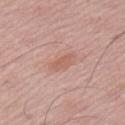The lesion was tiled from a total-body skin photograph and was not biopsied. Captured under white-light illumination. A close-up tile cropped from a whole-body skin photograph, about 15 mm across. A male patient, in their 50s. The lesion is located on the arm. Approximately 3 mm at its widest.